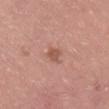This lesion was catalogued during total-body skin photography and was not selected for biopsy.
Located on the lower back.
This is a white-light tile.
The lesion's longest dimension is about 2.5 mm.
A 15 mm crop from a total-body photograph taken for skin-cancer surveillance.
A male subject about 40 years old.